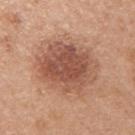The lesion was tiled from a total-body skin photograph and was not biopsied.
The tile uses white-light illumination.
A close-up tile cropped from a whole-body skin photograph, about 15 mm across.
The lesion is located on the arm.
A female patient in their 50s.
Longest diameter approximately 7.5 mm.